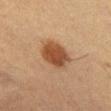Imaged during a routine full-body skin examination; the lesion was not biopsied and no histopathology is available.
This is a cross-polarized tile.
The patient is a female roughly 50 years of age.
Longest diameter approximately 4 mm.
A close-up tile cropped from a whole-body skin photograph, about 15 mm across.
The lesion is located on the left thigh.